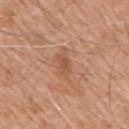Q: Was this lesion biopsied?
A: catalogued during a skin exam; not biopsied
Q: How was this image acquired?
A: 15 mm crop, total-body photography
Q: Who is the patient?
A: male, aged approximately 75
Q: What lighting was used for the tile?
A: white-light illumination
Q: Lesion location?
A: the arm
Q: What did automated image analysis measure?
A: an area of roughly 3 mm², an eccentricity of roughly 0.8, and a symmetry-axis asymmetry near 0.3; about 8 CIELAB-L* units darker than the surrounding skin and a normalized border contrast of about 5.5; peripheral color asymmetry of about 0.5; a nevus-likeness score of about 5/100 and a detector confidence of about 100 out of 100 that the crop contains a lesion